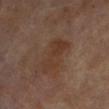Findings:
– biopsy status: total-body-photography surveillance lesion; no biopsy
– lighting: cross-polarized illumination
– site: the leg
– acquisition: ~15 mm tile from a whole-body skin photo
– subject: female, aged around 75